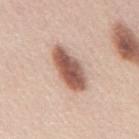notes: no biopsy performed (imaged during a skin exam); subject: male, aged approximately 45; imaging modality: total-body-photography crop, ~15 mm field of view; illumination: white-light illumination; body site: the back.